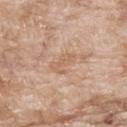notes: catalogued during a skin exam; not biopsied | image source: ~15 mm tile from a whole-body skin photo | lesion diameter: ≈3 mm | anatomic site: the upper back | image-analysis metrics: an area of roughly 4.5 mm², an eccentricity of roughly 0.85, and two-axis asymmetry of about 0.4; a lesion color around L≈61 a*≈18 b*≈31 in CIELAB and a normalized border contrast of about 4.5; a border-irregularity index near 4.5/10, a within-lesion color-variation index near 1/10, and radial color variation of about 0.5; lesion-presence confidence of about 100/100 | subject: male, in their mid- to late 60s | illumination: white-light.